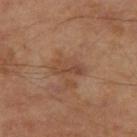Recorded during total-body skin imaging; not selected for excision or biopsy.
A male subject aged around 65.
A 15 mm close-up extracted from a 3D total-body photography capture.